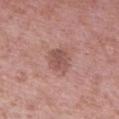Q: Is there a histopathology result?
A: no biopsy performed (imaged during a skin exam)
Q: What is the lesion's diameter?
A: ~3.5 mm (longest diameter)
Q: What is the anatomic site?
A: the right upper arm
Q: Patient demographics?
A: male, in their 40s
Q: What kind of image is this?
A: 15 mm crop, total-body photography
Q: Illumination type?
A: white-light illumination